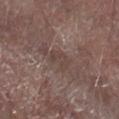Clinical impression:
This lesion was catalogued during total-body skin photography and was not selected for biopsy.
Context:
Located on the right forearm. This image is a 15 mm lesion crop taken from a total-body photograph. Automated image analysis of the tile measured a nevus-likeness score of about 0/100 and a lesion-detection confidence of about 80/100. The patient is a male roughly 65 years of age.